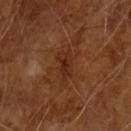| field | value |
|---|---|
| notes | imaged on a skin check; not biopsied |